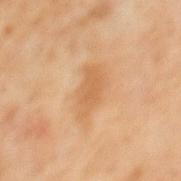Assessment:
Captured during whole-body skin photography for melanoma surveillance; the lesion was not biopsied.
Image and clinical context:
A 15 mm crop from a total-body photograph taken for skin-cancer surveillance. Automated image analysis of the tile measured a mean CIELAB color near L≈58 a*≈20 b*≈38, about 7 CIELAB-L* units darker than the surrounding skin, and a normalized border contrast of about 5.5. The software also gave a border-irregularity rating of about 3/10, a color-variation rating of about 1.5/10, and radial color variation of about 0.5. The software also gave lesion-presence confidence of about 100/100. Captured under cross-polarized illumination. The lesion is on the mid back. The subject is a male aged approximately 60. Approximately 4.5 mm at its widest.